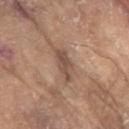Q: Was this lesion biopsied?
A: no biopsy performed (imaged during a skin exam)
Q: Who is the patient?
A: male, aged 78 to 82
Q: Lesion location?
A: the left upper arm
Q: What kind of image is this?
A: ~15 mm tile from a whole-body skin photo
Q: What lighting was used for the tile?
A: white-light illumination
Q: What is the lesion's diameter?
A: ≈4 mm